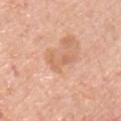  image:
    source: total-body photography crop
    field_of_view_mm: 15
  lesion_size:
    long_diameter_mm_approx: 3.0
  patient:
    sex: male
    age_approx: 50
  automated_metrics:
    cielab_L: 64
    cielab_a: 22
    cielab_b: 35
    vs_skin_darker_L: 8.0
    vs_skin_contrast_norm: 5.0
    nevus_likeness_0_100: 0
    lesion_detection_confidence_0_100: 100
  lighting: white-light
  site: right upper arm